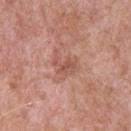Q: Was a biopsy performed?
A: total-body-photography surveillance lesion; no biopsy